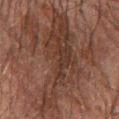| feature | finding |
|---|---|
| subject | male, aged approximately 70 |
| image source | total-body-photography crop, ~15 mm field of view |
| lighting | cross-polarized |
| image-analysis metrics | an area of roughly 37 mm²; a mean CIELAB color near L≈29 a*≈16 b*≈21 and about 7 CIELAB-L* units darker than the surrounding skin; internal color variation of about 4 on a 0–10 scale and peripheral color asymmetry of about 1.5 |
| location | the right forearm |